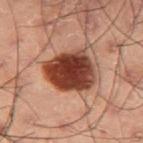<case>
<biopsy_status>not biopsied; imaged during a skin examination</biopsy_status>
<patient>
  <sex>male</sex>
  <age_approx>55</age_approx>
</patient>
<image>
  <source>total-body photography crop</source>
  <field_of_view_mm>15</field_of_view_mm>
</image>
<site>right thigh</site>
<lighting>cross-polarized</lighting>
</case>